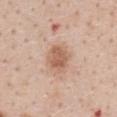Clinical summary:
A female subject, approximately 45 years of age. Cropped from a total-body skin-imaging series; the visible field is about 15 mm. The lesion is on the abdomen. Captured under white-light illumination. The lesion-visualizer software estimated a lesion area of about 9.5 mm², an outline eccentricity of about 0.6 (0 = round, 1 = elongated), and a shape-asymmetry score of about 0.15 (0 = symmetric). The software also gave an average lesion color of about L≈61 a*≈20 b*≈30 (CIELAB), a lesion–skin lightness drop of about 10, and a lesion-to-skin contrast of about 7 (normalized; higher = more distinct). And it measured an automated nevus-likeness rating near 55 out of 100. Approximately 4 mm at its widest.